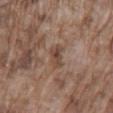The lesion is located on the lower back. A male patient, roughly 75 years of age. A close-up tile cropped from a whole-body skin photograph, about 15 mm across.